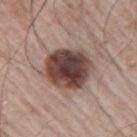Findings:
* follow-up — imaged on a skin check; not biopsied
* image — ~15 mm tile from a whole-body skin photo
* site — the right upper arm
* subject — male, roughly 65 years of age